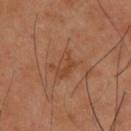| key | value |
|---|---|
| notes | catalogued during a skin exam; not biopsied |
| subject | male, approximately 40 years of age |
| size | ≈3 mm |
| tile lighting | cross-polarized illumination |
| image | 15 mm crop, total-body photography |
| image-analysis metrics | a lesion area of about 4.5 mm² and a shape eccentricity near 0.8; an average lesion color of about L≈43 a*≈22 b*≈33 (CIELAB) and a normalized border contrast of about 5; a border-irregularity index near 3.5/10, a color-variation rating of about 2.5/10, and radial color variation of about 1 |
| body site | the back |